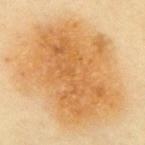Imaged during a routine full-body skin examination; the lesion was not biopsied and no histopathology is available. A female subject, roughly 60 years of age. From the mid back. Automated image analysis of the tile measured internal color variation of about 4.5 on a 0–10 scale and peripheral color asymmetry of about 1.5. A 15 mm close-up extracted from a 3D total-body photography capture.